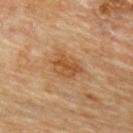The lesion was photographed on a routine skin check and not biopsied; there is no pathology result.
A male patient aged 83 or over.
About 4 mm across.
The lesion is located on the upper back.
A close-up tile cropped from a whole-body skin photograph, about 15 mm across.